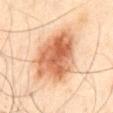Acquisition and patient details:
A male patient in their mid- to late 60s. A roughly 15 mm field-of-view crop from a total-body skin photograph. Measured at roughly 7 mm in maximum diameter. An algorithmic analysis of the crop reported a border-irregularity rating of about 2.5/10, a within-lesion color-variation index near 7/10, and a peripheral color-asymmetry measure near 2.5. The lesion is on the abdomen. Imaged with cross-polarized lighting.